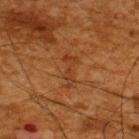This lesion was catalogued during total-body skin photography and was not selected for biopsy. Located on the upper back. A region of skin cropped from a whole-body photographic capture, roughly 15 mm wide. A male subject, roughly 65 years of age. Captured under cross-polarized illumination. The total-body-photography lesion software estimated a lesion area of about 4.5 mm², a shape eccentricity near 0.95, and a symmetry-axis asymmetry near 0.45. The software also gave border irregularity of about 6.5 on a 0–10 scale, a within-lesion color-variation index near 0.5/10, and a peripheral color-asymmetry measure near 0. And it measured a nevus-likeness score of about 0/100.